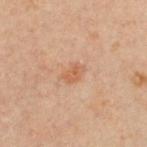Assessment: Recorded during total-body skin imaging; not selected for excision or biopsy. Clinical summary: A female subject, aged 28 to 32. This is a cross-polarized tile. The lesion is located on the front of the torso. Cropped from a whole-body photographic skin survey; the tile spans about 15 mm. Measured at roughly 2.5 mm in maximum diameter.